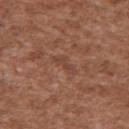biopsy_status: not biopsied; imaged during a skin examination
image:
  source: total-body photography crop
  field_of_view_mm: 15
automated_metrics:
  area_mm2_approx: 3.5
  cielab_L: 43
  cielab_a: 22
  cielab_b: 28
  vs_skin_darker_L: 6.0
  vs_skin_contrast_norm: 4.5
  border_irregularity_0_10: 5.5
  color_variation_0_10: 0.0
  peripheral_color_asymmetry: 0.0
  lesion_detection_confidence_0_100: 60
patient:
  sex: male
  age_approx: 45
site: back
lighting: white-light
lesion_size:
  long_diameter_mm_approx: 3.5